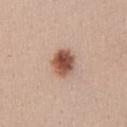Captured during whole-body skin photography for melanoma surveillance; the lesion was not biopsied. A female subject, about 30 years old. This image is a 15 mm lesion crop taken from a total-body photograph. The lesion is on the mid back.